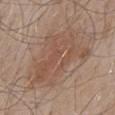Impression: The lesion was tiled from a total-body skin photograph and was not biopsied. Clinical summary: The subject is a male aged 53–57. Measured at roughly 9.5 mm in maximum diameter. On the mid back. Captured under white-light illumination. Cropped from a total-body skin-imaging series; the visible field is about 15 mm.